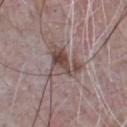Assessment: The lesion was photographed on a routine skin check and not biopsied; there is no pathology result. Image and clinical context: A roughly 15 mm field-of-view crop from a total-body skin photograph. The patient is a male in their mid-60s. On the chest.